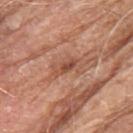Assessment:
The lesion was tiled from a total-body skin photograph and was not biopsied.
Clinical summary:
A close-up tile cropped from a whole-body skin photograph, about 15 mm across. From the upper back. The patient is a male aged 78–82. The lesion's longest dimension is about 3 mm. Imaged with white-light lighting.